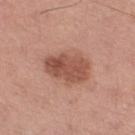Assessment:
The lesion was photographed on a routine skin check and not biopsied; there is no pathology result.
Acquisition and patient details:
This image is a 15 mm lesion crop taken from a total-body photograph. The subject is a male roughly 70 years of age. The lesion is located on the right thigh.